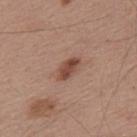{"biopsy_status": "not biopsied; imaged during a skin examination", "image": {"source": "total-body photography crop", "field_of_view_mm": 15}, "patient": {"sex": "male", "age_approx": 55}, "lighting": "white-light", "site": "back", "lesion_size": {"long_diameter_mm_approx": 3.5}}